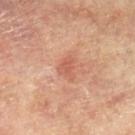This lesion was catalogued during total-body skin photography and was not selected for biopsy. A 15 mm close-up extracted from a 3D total-body photography capture. Imaged with cross-polarized lighting. A female patient about 75 years old. The lesion is located on the left lower leg. The lesion's longest dimension is about 3 mm.